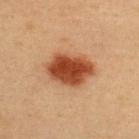Q: Was a biopsy performed?
A: imaged on a skin check; not biopsied
Q: Lesion location?
A: the upper back
Q: Automated lesion metrics?
A: a mean CIELAB color near L≈42 a*≈26 b*≈34, about 16 CIELAB-L* units darker than the surrounding skin, and a normalized lesion–skin contrast near 12; a border-irregularity index near 2/10 and internal color variation of about 4.5 on a 0–10 scale
Q: What kind of image is this?
A: ~15 mm crop, total-body skin-cancer survey
Q: Illumination type?
A: cross-polarized
Q: Who is the patient?
A: male, roughly 40 years of age
Q: What is the lesion's diameter?
A: ~5.5 mm (longest diameter)